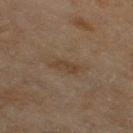<lesion>
  <biopsy_status>not biopsied; imaged during a skin examination</biopsy_status>
  <image>
    <source>total-body photography crop</source>
    <field_of_view_mm>15</field_of_view_mm>
  </image>
  <patient>
    <sex>female</sex>
    <age_approx>60</age_approx>
  </patient>
  <site>leg</site>
</lesion>